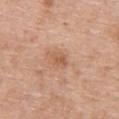Assessment:
Captured during whole-body skin photography for melanoma surveillance; the lesion was not biopsied.
Clinical summary:
A 15 mm close-up tile from a total-body photography series done for melanoma screening. A male subject, approximately 65 years of age. The tile uses white-light illumination. The lesion-visualizer software estimated a lesion color around L≈57 a*≈22 b*≈32 in CIELAB, about 9 CIELAB-L* units darker than the surrounding skin, and a normalized border contrast of about 6. The software also gave a border-irregularity rating of about 2/10 and radial color variation of about 0.5. It also reported a classifier nevus-likeness of about 0/100 and a detector confidence of about 100 out of 100 that the crop contains a lesion. The lesion is located on the upper back.